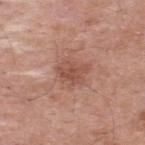Clinical impression: Imaged during a routine full-body skin examination; the lesion was not biopsied and no histopathology is available. Clinical summary: A male patient about 55 years old. The lesion is on the upper back. Captured under white-light illumination. This image is a 15 mm lesion crop taken from a total-body photograph.